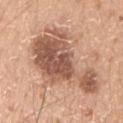Recorded during total-body skin imaging; not selected for excision or biopsy.
A 15 mm crop from a total-body photograph taken for skin-cancer surveillance.
About 9 mm across.
On the left upper arm.
The tile uses white-light illumination.
A male subject aged around 20.